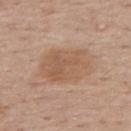Q: Is there a histopathology result?
A: imaged on a skin check; not biopsied
Q: What is the imaging modality?
A: total-body-photography crop, ~15 mm field of view
Q: How was the tile lit?
A: white-light illumination
Q: Lesion size?
A: about 5.5 mm
Q: What are the patient's age and sex?
A: female, aged approximately 65
Q: What did automated image analysis measure?
A: an area of roughly 19 mm² and two-axis asymmetry of about 0.15; roughly 8 lightness units darker than nearby skin and a normalized border contrast of about 6; a classifier nevus-likeness of about 0/100 and a lesion-detection confidence of about 100/100
Q: Where on the body is the lesion?
A: the upper back